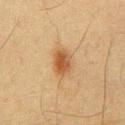notes: no biopsy performed (imaged during a skin exam) | patient: male, aged 58 to 62 | body site: the chest | image-analysis metrics: a normalized lesion–skin contrast near 8.5; a border-irregularity rating of about 2/10, internal color variation of about 4 on a 0–10 scale, and peripheral color asymmetry of about 1.5; a classifier nevus-likeness of about 100/100 and a detector confidence of about 100 out of 100 that the crop contains a lesion | tile lighting: cross-polarized | imaging modality: ~15 mm tile from a whole-body skin photo | diameter: ≈3.5 mm.